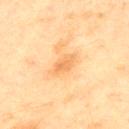{
  "biopsy_status": "not biopsied; imaged during a skin examination",
  "lighting": "cross-polarized",
  "lesion_size": {
    "long_diameter_mm_approx": 3.5
  },
  "site": "upper back",
  "image": {
    "source": "total-body photography crop",
    "field_of_view_mm": 15
  },
  "patient": {
    "sex": "male",
    "age_approx": 45
  }
}